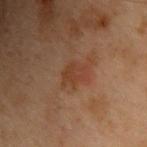Imaged during a routine full-body skin examination; the lesion was not biopsied and no histopathology is available. The lesion is located on the upper back. Measured at roughly 2.5 mm in maximum diameter. A male patient approximately 55 years of age. Imaged with cross-polarized lighting. Cropped from a total-body skin-imaging series; the visible field is about 15 mm. Automated tile analysis of the lesion measured a footprint of about 4.5 mm², a shape eccentricity near 0.6, and a shape-asymmetry score of about 0.25 (0 = symmetric). And it measured a mean CIELAB color near L≈31 a*≈19 b*≈26, roughly 5 lightness units darker than nearby skin, and a normalized lesion–skin contrast near 5.5. The analysis additionally found a border-irregularity index near 2.5/10 and a peripheral color-asymmetry measure near 1.